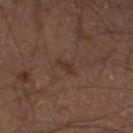image: ~15 mm tile from a whole-body skin photo
size: ≈2 mm
automated lesion analysis: border irregularity of about 3 on a 0–10 scale, a within-lesion color-variation index near 0/10, and a peripheral color-asymmetry measure near 0; an automated nevus-likeness rating near 0 out of 100
subject: male, aged 58 to 62
body site: the left thigh
tile lighting: cross-polarized illumination